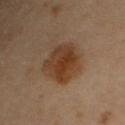image source=total-body-photography crop, ~15 mm field of view
location=the upper back
diameter=~6 mm (longest diameter)
patient=female, about 60 years old